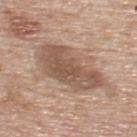| feature | finding |
|---|---|
| site | the upper back |
| automated metrics | a mean CIELAB color near L≈54 a*≈17 b*≈27 and roughly 11 lightness units darker than nearby skin; a nevus-likeness score of about 10/100 and a detector confidence of about 100 out of 100 that the crop contains a lesion |
| tile lighting | white-light |
| patient | male, aged 73–77 |
| image source | 15 mm crop, total-body photography |